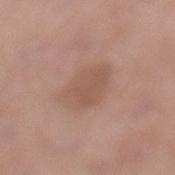The lesion was photographed on a routine skin check and not biopsied; there is no pathology result. The lesion's longest dimension is about 5.5 mm. Captured under white-light illumination. A female subject, about 65 years old. Cropped from a total-body skin-imaging series; the visible field is about 15 mm. On the left lower leg.